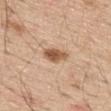Longest diameter approximately 3.5 mm. The subject is a male about 70 years old. This is a white-light tile. A 15 mm crop from a total-body photograph taken for skin-cancer surveillance. From the back.